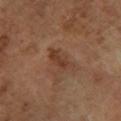Notes:
– workup — no biopsy performed (imaged during a skin exam)
– TBP lesion metrics — a nevus-likeness score of about 5/100
– body site — the right lower leg
– subject — female, aged 63–67
– illumination — cross-polarized illumination
– diameter — ≈4 mm
– image source — ~15 mm crop, total-body skin-cancer survey